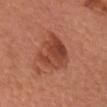follow-up: catalogued during a skin exam; not biopsied
imaging modality: ~15 mm tile from a whole-body skin photo
size: about 5 mm
anatomic site: the front of the torso
patient: female, aged 63 to 67